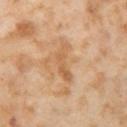Assessment:
Recorded during total-body skin imaging; not selected for excision or biopsy.
Context:
A 15 mm crop from a total-body photograph taken for skin-cancer surveillance. Approximately 5 mm at its widest. The total-body-photography lesion software estimated a mean CIELAB color near L≈61 a*≈21 b*≈38 and a lesion-to-skin contrast of about 6 (normalized; higher = more distinct). The analysis additionally found border irregularity of about 7 on a 0–10 scale, internal color variation of about 2 on a 0–10 scale, and a peripheral color-asymmetry measure near 0.5. The lesion is located on the left thigh. A female subject, aged 53 to 57.